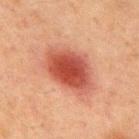workup: total-body-photography surveillance lesion; no biopsy
size: ≈6.5 mm
patient: male, roughly 65 years of age
illumination: cross-polarized
image: ~15 mm tile from a whole-body skin photo
TBP lesion metrics: a footprint of about 20 mm²; a lesion color around L≈41 a*≈27 b*≈28 in CIELAB, about 13 CIELAB-L* units darker than the surrounding skin, and a lesion-to-skin contrast of about 10 (normalized; higher = more distinct); a lesion-detection confidence of about 100/100
body site: the chest